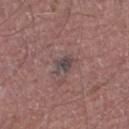Part of a total-body skin-imaging series; this lesion was reviewed on a skin check and was not flagged for biopsy.